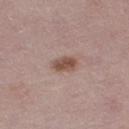No biopsy was performed on this lesion — it was imaged during a full skin examination and was not determined to be concerning.
A female subject roughly 50 years of age.
The lesion is on the right thigh.
Automated tile analysis of the lesion measured a lesion area of about 4.5 mm² and an eccentricity of roughly 0.85. It also reported an average lesion color of about L≈50 a*≈19 b*≈25 (CIELAB) and a normalized border contrast of about 9. It also reported border irregularity of about 2.5 on a 0–10 scale, a color-variation rating of about 2.5/10, and peripheral color asymmetry of about 1. And it measured a classifier nevus-likeness of about 90/100 and lesion-presence confidence of about 100/100.
A 15 mm close-up extracted from a 3D total-body photography capture.
Imaged with white-light lighting.
Measured at roughly 3 mm in maximum diameter.